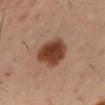A male patient, aged approximately 40.
Cropped from a total-body skin-imaging series; the visible field is about 15 mm.
On the back.